Measured at roughly 2 mm in maximum diameter · Automated image analysis of the tile measured a footprint of about 2.5 mm², a shape eccentricity near 0.5, and a shape-asymmetry score of about 0.25 (0 = symmetric). And it measured a classifier nevus-likeness of about 100/100 and lesion-presence confidence of about 100/100 · on the mid back · the patient is a female aged 38–42 · the tile uses cross-polarized illumination · a close-up tile cropped from a whole-body skin photograph, about 15 mm across — 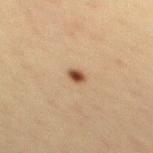biopsy diagnosis: a melanocytic nevus — a benign skin lesion.